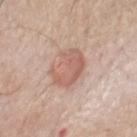Part of a total-body skin-imaging series; this lesion was reviewed on a skin check and was not flagged for biopsy.
This is a white-light tile.
Measured at roughly 5 mm in maximum diameter.
On the front of the torso.
A male subject, aged around 65.
Cropped from a whole-body photographic skin survey; the tile spans about 15 mm.